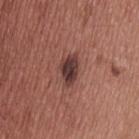| feature | finding |
|---|---|
| workup | imaged on a skin check; not biopsied |
| lesion diameter | ~3.5 mm (longest diameter) |
| acquisition | total-body-photography crop, ~15 mm field of view |
| automated metrics | a mean CIELAB color near L≈37 a*≈21 b*≈20, about 13 CIELAB-L* units darker than the surrounding skin, and a normalized border contrast of about 11.5; a border-irregularity index near 3/10 and a color-variation rating of about 4/10; a lesion-detection confidence of about 100/100 |
| anatomic site | the upper back |
| subject | male, aged 38–42 |
| tile lighting | white-light |